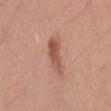Impression: The lesion was photographed on a routine skin check and not biopsied; there is no pathology result. Image and clinical context: A roughly 15 mm field-of-view crop from a total-body skin photograph. On the back. The recorded lesion diameter is about 5 mm. The lesion-visualizer software estimated a lesion area of about 6.5 mm², an eccentricity of roughly 0.95, and a shape-asymmetry score of about 0.3 (0 = symmetric). It also reported a border-irregularity index near 4/10 and a color-variation rating of about 3.5/10. It also reported a classifier nevus-likeness of about 60/100. A male subject, aged 28–32. Imaged with white-light lighting.